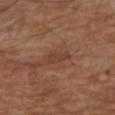Notes:
- acquisition — ~15 mm tile from a whole-body skin photo
- image-analysis metrics — an eccentricity of roughly 0.9
- subject — male, aged around 75
- location — the upper back